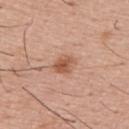Q: Patient demographics?
A: male, in their mid- to late 50s
Q: Automated lesion metrics?
A: a mean CIELAB color near L≈56 a*≈23 b*≈32, roughly 11 lightness units darker than nearby skin, and a normalized lesion–skin contrast near 8
Q: Lesion size?
A: ≈3 mm
Q: Illumination type?
A: white-light illumination
Q: How was this image acquired?
A: total-body-photography crop, ~15 mm field of view